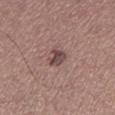{
  "biopsy_status": "not biopsied; imaged during a skin examination",
  "site": "left lower leg",
  "patient": {
    "sex": "male",
    "age_approx": 40
  },
  "image": {
    "source": "total-body photography crop",
    "field_of_view_mm": 15
  }
}